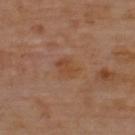No biopsy was performed on this lesion — it was imaged during a full skin examination and was not determined to be concerning.
On the upper back.
Measured at roughly 3 mm in maximum diameter.
Cropped from a whole-body photographic skin survey; the tile spans about 15 mm.
Captured under cross-polarized illumination.